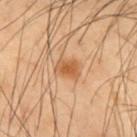workup: total-body-photography surveillance lesion; no biopsy
image: total-body-photography crop, ~15 mm field of view
diameter: ~3 mm (longest diameter)
location: the chest
tile lighting: cross-polarized
image-analysis metrics: an outline eccentricity of about 0.7 (0 = round, 1 = elongated) and a symmetry-axis asymmetry near 0.2; a mean CIELAB color near L≈46 a*≈20 b*≈33
subject: male, in their mid-50s